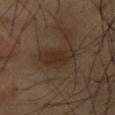workup — no biopsy performed (imaged during a skin exam)